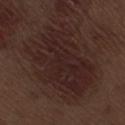workup: imaged on a skin check; not biopsied
subject: male, aged 68–72
location: the right thigh
automated lesion analysis: a lesion-to-skin contrast of about 8 (normalized; higher = more distinct); a border-irregularity rating of about 5.5/10, internal color variation of about 3 on a 0–10 scale, and a peripheral color-asymmetry measure near 1
image: total-body-photography crop, ~15 mm field of view
diameter: about 9.5 mm